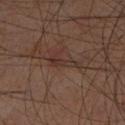| feature | finding |
|---|---|
| biopsy status | no biopsy performed (imaged during a skin exam) |
| image | 15 mm crop, total-body photography |
| illumination | cross-polarized |
| diameter | ≈3.5 mm |
| site | the leg |
| automated metrics | a mean CIELAB color near L≈27 a*≈15 b*≈20; an automated nevus-likeness rating near 0 out of 100 and a lesion-detection confidence of about 75/100 |
| subject | male, aged 58 to 62 |